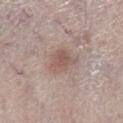The lesion was photographed on a routine skin check and not biopsied; there is no pathology result.
Imaged with white-light lighting.
Approximately 3.5 mm at its widest.
The lesion is on the left lower leg.
The subject is a male aged 78–82.
A lesion tile, about 15 mm wide, cut from a 3D total-body photograph.